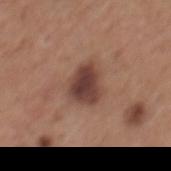Q: Was this lesion biopsied?
A: catalogued during a skin exam; not biopsied
Q: Patient demographics?
A: male, aged approximately 65
Q: What is the lesion's diameter?
A: ~4 mm (longest diameter)
Q: How was the tile lit?
A: white-light illumination
Q: How was this image acquired?
A: ~15 mm tile from a whole-body skin photo
Q: Where on the body is the lesion?
A: the mid back
Q: Automated lesion metrics?
A: a lesion area of about 11 mm² and a shape-asymmetry score of about 0.3 (0 = symmetric); border irregularity of about 2.5 on a 0–10 scale and internal color variation of about 4.5 on a 0–10 scale; a classifier nevus-likeness of about 75/100 and a detector confidence of about 100 out of 100 that the crop contains a lesion